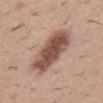<case>
  <biopsy_status>not biopsied; imaged during a skin examination</biopsy_status>
  <lighting>white-light</lighting>
  <image>
    <source>total-body photography crop</source>
    <field_of_view_mm>15</field_of_view_mm>
  </image>
  <lesion_size>
    <long_diameter_mm_approx>7.5</long_diameter_mm_approx>
  </lesion_size>
  <automated_metrics>
    <cielab_L>51</cielab_L>
    <cielab_a>20</cielab_a>
    <cielab_b>25</cielab_b>
    <vs_skin_darker_L>16.0</vs_skin_darker_L>
    <vs_skin_contrast_norm>10.5</vs_skin_contrast_norm>
  </automated_metrics>
  <patient>
    <sex>male</sex>
    <age_approx>30</age_approx>
  </patient>
  <site>abdomen</site>
</case>